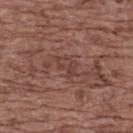  patient:
    sex: female
    age_approx: 75
  lighting: white-light
  image:
    source: total-body photography crop
    field_of_view_mm: 15
  site: upper back
  automated_metrics:
    cielab_L: 41
    cielab_a: 21
    cielab_b: 23
    vs_skin_contrast_norm: 5.0
    nevus_likeness_0_100: 0
    lesion_detection_confidence_0_100: 80